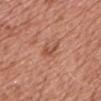<case>
<biopsy_status>not biopsied; imaged during a skin examination</biopsy_status>
<image>
  <source>total-body photography crop</source>
  <field_of_view_mm>15</field_of_view_mm>
</image>
<site>chest</site>
<patient>
  <sex>male</sex>
  <age_approx>65</age_approx>
</patient>
</case>